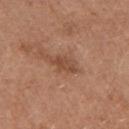Clinical impression: Imaged during a routine full-body skin examination; the lesion was not biopsied and no histopathology is available. Image and clinical context: The lesion is located on the left upper arm. A male subject, roughly 75 years of age. A 15 mm close-up extracted from a 3D total-body photography capture. Captured under white-light illumination. About 3.5 mm across.